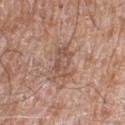Q: Is there a histopathology result?
A: imaged on a skin check; not biopsied
Q: Automated lesion metrics?
A: an outline eccentricity of about 0.9 (0 = round, 1 = elongated); a classifier nevus-likeness of about 0/100 and lesion-presence confidence of about 90/100
Q: What lighting was used for the tile?
A: white-light illumination
Q: Lesion location?
A: the right thigh
Q: What are the patient's age and sex?
A: male, aged 58–62
Q: Lesion size?
A: about 5 mm
Q: What kind of image is this?
A: ~15 mm tile from a whole-body skin photo